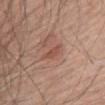Recorded during total-body skin imaging; not selected for excision or biopsy. A region of skin cropped from a whole-body photographic capture, roughly 15 mm wide. From the front of the torso. A male subject, aged 78 to 82.